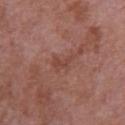Assessment:
Recorded during total-body skin imaging; not selected for excision or biopsy.
Background:
The subject is a female in their 70s. The lesion is on the right upper arm. The lesion's longest dimension is about 3 mm. A roughly 15 mm field-of-view crop from a total-body skin photograph. The total-body-photography lesion software estimated a footprint of about 3 mm², a shape eccentricity near 0.9, and a symmetry-axis asymmetry near 0.55. It also reported a mean CIELAB color near L≈44 a*≈24 b*≈26, a lesion–skin lightness drop of about 7, and a normalized border contrast of about 5.5. And it measured an automated nevus-likeness rating near 0 out of 100 and a lesion-detection confidence of about 100/100.